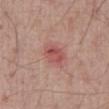Impression:
Imaged during a routine full-body skin examination; the lesion was not biopsied and no histopathology is available.
Acquisition and patient details:
Approximately 3 mm at its widest. The subject is a male aged 63 to 67. From the front of the torso. Cropped from a whole-body photographic skin survey; the tile spans about 15 mm.